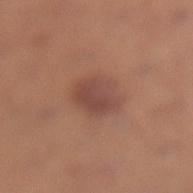Clinical impression:
Imaged during a routine full-body skin examination; the lesion was not biopsied and no histopathology is available.
Background:
Captured under white-light illumination. A 15 mm crop from a total-body photograph taken for skin-cancer surveillance. Automated image analysis of the tile measured an eccentricity of roughly 0.7 and a symmetry-axis asymmetry near 0.2. And it measured a border-irregularity rating of about 2/10 and radial color variation of about 1. And it measured an automated nevus-likeness rating near 70 out of 100 and a lesion-detection confidence of about 100/100. A female subject aged around 35. From the right lower leg.